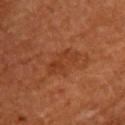{
  "biopsy_status": "not biopsied; imaged during a skin examination",
  "image": {
    "source": "total-body photography crop",
    "field_of_view_mm": 15
  },
  "site": "upper back",
  "automated_metrics": {
    "area_mm2_approx": 7.5,
    "eccentricity": 0.9,
    "shape_asymmetry": 0.3,
    "nevus_likeness_0_100": 10
  },
  "patient": {
    "sex": "female",
    "age_approx": 65
  },
  "lesion_size": {
    "long_diameter_mm_approx": 4.5
  }
}